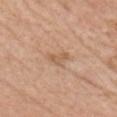subject = female, approximately 70 years of age | acquisition = 15 mm crop, total-body photography | diameter = ~2.5 mm (longest diameter) | location = the front of the torso | illumination = white-light.